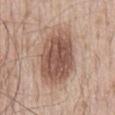biopsy status=no biopsy performed (imaged during a skin exam)
image=~15 mm crop, total-body skin-cancer survey
TBP lesion metrics=an average lesion color of about L≈53 a*≈19 b*≈26 (CIELAB) and a normalized border contrast of about 9.5; a border-irregularity index near 2/10, a within-lesion color-variation index near 5/10, and peripheral color asymmetry of about 1.5; an automated nevus-likeness rating near 95 out of 100
diameter=about 7.5 mm
anatomic site=the back
subject=male, in their 50s
lighting=white-light illumination